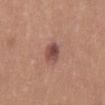{
  "biopsy_status": "not biopsied; imaged during a skin examination",
  "patient": {
    "sex": "male",
    "age_approx": 25
  },
  "site": "abdomen",
  "lesion_size": {
    "long_diameter_mm_approx": 3.0
  },
  "image": {
    "source": "total-body photography crop",
    "field_of_view_mm": 15
  }
}